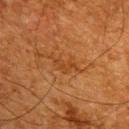<lesion>
  <biopsy_status>not biopsied; imaged during a skin examination</biopsy_status>
  <patient>
    <sex>male</sex>
    <age_approx>65</age_approx>
  </patient>
  <site>upper back</site>
  <image>
    <source>total-body photography crop</source>
    <field_of_view_mm>15</field_of_view_mm>
  </image>
</lesion>